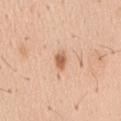No biopsy was performed on this lesion — it was imaged during a full skin examination and was not determined to be concerning.
On the right upper arm.
A close-up tile cropped from a whole-body skin photograph, about 15 mm across.
This is a white-light tile.
A male subject aged approximately 40.